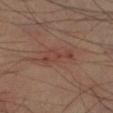{"site": "left lower leg", "image": {"source": "total-body photography crop", "field_of_view_mm": 15}, "patient": {"sex": "male", "age_approx": 40}}